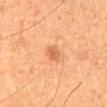Q: Is there a histopathology result?
A: imaged on a skin check; not biopsied
Q: How was this image acquired?
A: ~15 mm tile from a whole-body skin photo
Q: What are the patient's age and sex?
A: male, roughly 60 years of age
Q: What lighting was used for the tile?
A: cross-polarized illumination
Q: Lesion location?
A: the back
Q: How large is the lesion?
A: about 2.5 mm